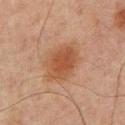Imaged during a routine full-body skin examination; the lesion was not biopsied and no histopathology is available. A 15 mm close-up extracted from a 3D total-body photography capture. From the upper back. The lesion's longest dimension is about 5 mm. The total-body-photography lesion software estimated an area of roughly 13 mm², an eccentricity of roughly 0.65, and a symmetry-axis asymmetry near 0.15. And it measured a lesion color around L≈40 a*≈20 b*≈29 in CIELAB, a lesion–skin lightness drop of about 8, and a lesion-to-skin contrast of about 7.5 (normalized; higher = more distinct). The software also gave internal color variation of about 3 on a 0–10 scale and a peripheral color-asymmetry measure near 1. This is a cross-polarized tile. A male patient, about 65 years old.